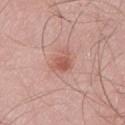The lesion was photographed on a routine skin check and not biopsied; there is no pathology result.
A male subject roughly 55 years of age.
This is a white-light tile.
This image is a 15 mm lesion crop taken from a total-body photograph.
The lesion's longest dimension is about 3 mm.
From the left thigh.